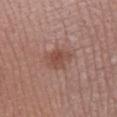Background: From the right lower leg. A male patient, aged approximately 50. About 3.5 mm across. An algorithmic analysis of the crop reported an average lesion color of about L≈48 a*≈22 b*≈25 (CIELAB), a lesion–skin lightness drop of about 8, and a normalized border contrast of about 6.5. And it measured a border-irregularity index near 2.5/10, internal color variation of about 3.5 on a 0–10 scale, and a peripheral color-asymmetry measure near 1. The analysis additionally found a lesion-detection confidence of about 100/100. Imaged with white-light lighting. A 15 mm close-up tile from a total-body photography series done for melanoma screening.